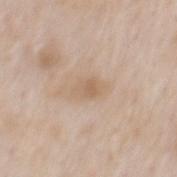No biopsy was performed on this lesion — it was imaged during a full skin examination and was not determined to be concerning.